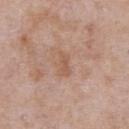• notes: catalogued during a skin exam; not biopsied
• patient: male, in their mid-50s
• anatomic site: the chest
• imaging modality: ~15 mm tile from a whole-body skin photo